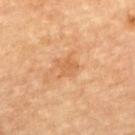<lesion>
<biopsy_status>not biopsied; imaged during a skin examination</biopsy_status>
<lighting>cross-polarized</lighting>
<patient>
  <sex>male</sex>
  <age_approx>85+</age_approx>
</patient>
<image>
  <source>total-body photography crop</source>
  <field_of_view_mm>15</field_of_view_mm>
</image>
<site>upper back</site>
<lesion_size>
  <long_diameter_mm_approx>2.5</long_diameter_mm_approx>
</lesion_size>
</lesion>